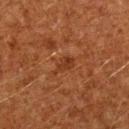Notes:
* image-analysis metrics · a footprint of about 3.5 mm², a shape eccentricity near 0.85, and a symmetry-axis asymmetry near 0.35
* subject · male, approximately 60 years of age
* location · the arm
* acquisition · total-body-photography crop, ~15 mm field of view
* lesion size · about 3 mm
* illumination · cross-polarized illumination